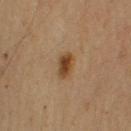A close-up tile cropped from a whole-body skin photograph, about 15 mm across.
A male subject, about 60 years old.
Located on the right upper arm.
Captured under cross-polarized illumination.
Longest diameter approximately 3 mm.